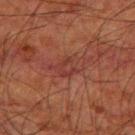Impression:
Part of a total-body skin-imaging series; this lesion was reviewed on a skin check and was not flagged for biopsy.
Clinical summary:
The lesion is located on the leg. A male patient approximately 80 years of age. A 15 mm close-up extracted from a 3D total-body photography capture. The lesion's longest dimension is about 4 mm. Imaged with cross-polarized lighting.